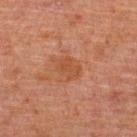| key | value |
|---|---|
| biopsy status | no biopsy performed (imaged during a skin exam) |
| image source | ~15 mm crop, total-body skin-cancer survey |
| location | the upper back |
| tile lighting | cross-polarized illumination |
| patient | male, approximately 60 years of age |